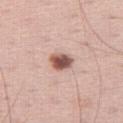Clinical impression:
Imaged during a routine full-body skin examination; the lesion was not biopsied and no histopathology is available.
Image and clinical context:
Longest diameter approximately 3 mm. A 15 mm crop from a total-body photograph taken for skin-cancer surveillance. An algorithmic analysis of the crop reported an average lesion color of about L≈54 a*≈21 b*≈25 (CIELAB), a lesion–skin lightness drop of about 18, and a normalized lesion–skin contrast near 11.5. The software also gave internal color variation of about 5.5 on a 0–10 scale and peripheral color asymmetry of about 1.5. The patient is a male in their 60s. The lesion is located on the leg. This is a white-light tile.